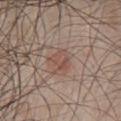workup: imaged on a skin check; not biopsied
image: total-body-photography crop, ~15 mm field of view
patient: male, in their 50s
illumination: white-light illumination
diameter: about 3 mm
automated lesion analysis: a footprint of about 5 mm², a shape eccentricity near 0.6, and a shape-asymmetry score of about 0.3 (0 = symmetric); a mean CIELAB color near L≈50 a*≈19 b*≈24, a lesion–skin lightness drop of about 7, and a lesion-to-skin contrast of about 5.5 (normalized; higher = more distinct); internal color variation of about 3.5 on a 0–10 scale; a detector confidence of about 100 out of 100 that the crop contains a lesion
site: the abdomen